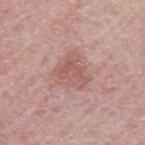This lesion was catalogued during total-body skin photography and was not selected for biopsy.
A female patient, about 65 years old.
The total-body-photography lesion software estimated a lesion area of about 8.5 mm², an outline eccentricity of about 0.55 (0 = round, 1 = elongated), and two-axis asymmetry of about 0.45. The software also gave a lesion color around L≈56 a*≈23 b*≈24 in CIELAB and about 8 CIELAB-L* units darker than the surrounding skin.
Captured under white-light illumination.
Located on the arm.
The recorded lesion diameter is about 4 mm.
A roughly 15 mm field-of-view crop from a total-body skin photograph.